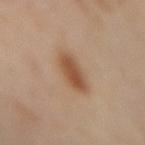{
  "biopsy_status": "not biopsied; imaged during a skin examination",
  "lesion_size": {
    "long_diameter_mm_approx": 4.5
  },
  "patient": {
    "sex": "female",
    "age_approx": 55
  },
  "site": "mid back",
  "image": {
    "source": "total-body photography crop",
    "field_of_view_mm": 15
  },
  "automated_metrics": {
    "border_irregularity_0_10": 2.0,
    "color_variation_0_10": 3.0
  },
  "lighting": "cross-polarized"
}